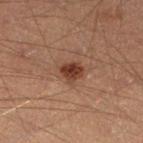The lesion was photographed on a routine skin check and not biopsied; there is no pathology result.
The subject is a male roughly 40 years of age.
Captured under cross-polarized illumination.
The lesion is located on the left lower leg.
Longest diameter approximately 3 mm.
A 15 mm crop from a total-body photograph taken for skin-cancer surveillance.